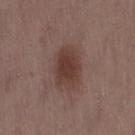Findings:
* notes — catalogued during a skin exam; not biopsied
* lighting — white-light illumination
* subject — female, roughly 50 years of age
* site — the right thigh
* size — ≈4 mm
* acquisition — ~15 mm tile from a whole-body skin photo